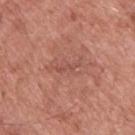Impression: No biopsy was performed on this lesion — it was imaged during a full skin examination and was not determined to be concerning. Image and clinical context: The lesion's longest dimension is about 3 mm. A male subject, roughly 55 years of age. Captured under white-light illumination. This image is a 15 mm lesion crop taken from a total-body photograph. On the upper back.